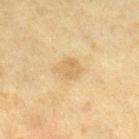Assessment: The lesion was tiled from a total-body skin photograph and was not biopsied. Background: Imaged with cross-polarized lighting. A female patient aged approximately 55. The total-body-photography lesion software estimated a lesion–skin lightness drop of about 7 and a normalized border contrast of about 5. The analysis additionally found border irregularity of about 1.5 on a 0–10 scale and internal color variation of about 1.5 on a 0–10 scale. The software also gave a lesion-detection confidence of about 100/100. The lesion is located on the right lower leg. Cropped from a whole-body photographic skin survey; the tile spans about 15 mm.